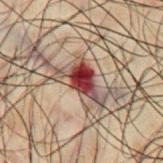<lesion>
  <biopsy_status>not biopsied; imaged during a skin examination</biopsy_status>
  <patient>
    <sex>male</sex>
    <age_approx>50</age_approx>
  </patient>
  <lighting>cross-polarized</lighting>
  <site>abdomen</site>
  <lesion_size>
    <long_diameter_mm_approx>5.5</long_diameter_mm_approx>
  </lesion_size>
  <image>
    <source>total-body photography crop</source>
    <field_of_view_mm>15</field_of_view_mm>
  </image>
</lesion>